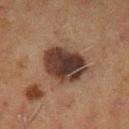Impression:
Imaged during a routine full-body skin examination; the lesion was not biopsied and no histopathology is available.
Background:
The lesion-visualizer software estimated a border-irregularity index near 1.5/10, internal color variation of about 5.5 on a 0–10 scale, and a peripheral color-asymmetry measure near 1.5. The analysis additionally found a nevus-likeness score of about 15/100. The lesion's longest dimension is about 5.5 mm. The lesion is on the left lower leg. A 15 mm close-up extracted from a 3D total-body photography capture. This is a cross-polarized tile. The patient is a female roughly 50 years of age.